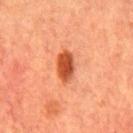Q: Is there a histopathology result?
A: catalogued during a skin exam; not biopsied
Q: Illumination type?
A: cross-polarized illumination
Q: How was this image acquired?
A: ~15 mm crop, total-body skin-cancer survey
Q: What is the anatomic site?
A: the chest
Q: Patient demographics?
A: male, in their mid-60s
Q: What is the lesion's diameter?
A: ≈4 mm
Q: What did automated image analysis measure?
A: a lesion–skin lightness drop of about 15 and a lesion-to-skin contrast of about 10.5 (normalized; higher = more distinct); a classifier nevus-likeness of about 100/100 and a detector confidence of about 100 out of 100 that the crop contains a lesion